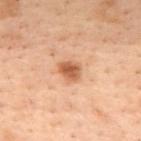{
  "biopsy_status": "not biopsied; imaged during a skin examination",
  "lighting": "cross-polarized",
  "site": "mid back",
  "image": {
    "source": "total-body photography crop",
    "field_of_view_mm": 15
  },
  "lesion_size": {
    "long_diameter_mm_approx": 2.5
  },
  "patient": {
    "sex": "female",
    "age_approx": 55
  }
}